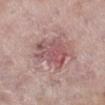Q: Was a biopsy performed?
A: no biopsy performed (imaged during a skin exam)
Q: What is the imaging modality?
A: total-body-photography crop, ~15 mm field of view
Q: What lighting was used for the tile?
A: white-light illumination
Q: Lesion location?
A: the right lower leg
Q: Automated lesion metrics?
A: a lesion area of about 14 mm², an eccentricity of roughly 0.75, and two-axis asymmetry of about 0.35; a mean CIELAB color near L≈53 a*≈23 b*≈19 and a normalized border contrast of about 6.5; an automated nevus-likeness rating near 0 out of 100 and a detector confidence of about 100 out of 100 that the crop contains a lesion
Q: Who is the patient?
A: female, about 70 years old